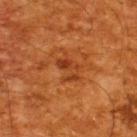Clinical impression: The lesion was tiled from a total-body skin photograph and was not biopsied. Context: A male subject aged 58 to 62. A 15 mm close-up tile from a total-body photography series done for melanoma screening. On the upper back.